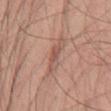This lesion was catalogued during total-body skin photography and was not selected for biopsy. The subject is a male aged approximately 60. Approximately 4 mm at its widest. The total-body-photography lesion software estimated a lesion area of about 6 mm², an eccentricity of roughly 0.9, and a shape-asymmetry score of about 0.35 (0 = symmetric). The software also gave a lesion color around L≈55 a*≈21 b*≈26 in CIELAB and a lesion-to-skin contrast of about 6 (normalized; higher = more distinct). It also reported a border-irregularity index near 4/10, a color-variation rating of about 4/10, and radial color variation of about 1. The lesion is located on the mid back. A close-up tile cropped from a whole-body skin photograph, about 15 mm across. Imaged with white-light lighting.